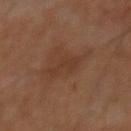Impression:
No biopsy was performed on this lesion — it was imaged during a full skin examination and was not determined to be concerning.
Image and clinical context:
Captured under cross-polarized illumination. A male patient, in their 60s. A 15 mm close-up extracted from a 3D total-body photography capture. The lesion is on the mid back. The lesion-visualizer software estimated two-axis asymmetry of about 0.5. And it measured roughly 5 lightness units darker than nearby skin. The software also gave a border-irregularity rating of about 6/10, internal color variation of about 1 on a 0–10 scale, and a peripheral color-asymmetry measure near 0. Approximately 3.5 mm at its widest.